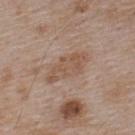workup=catalogued during a skin exam; not biopsied | subject=male, aged 53 to 57 | image=15 mm crop, total-body photography | anatomic site=the upper back.